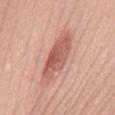Q: Is there a histopathology result?
A: total-body-photography surveillance lesion; no biopsy
Q: Lesion size?
A: ~8 mm (longest diameter)
Q: Lesion location?
A: the mid back
Q: How was this image acquired?
A: ~15 mm crop, total-body skin-cancer survey
Q: Illumination type?
A: white-light illumination
Q: What are the patient's age and sex?
A: female, aged 38 to 42